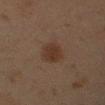notes: imaged on a skin check; not biopsied | location: the left upper arm | acquisition: 15 mm crop, total-body photography | patient: male, aged 43–47.